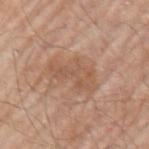Findings:
- biopsy status · catalogued during a skin exam; not biopsied
- lesion size · ≈5.5 mm
- body site · the arm
- subject · male, aged 63 to 67
- image source · ~15 mm tile from a whole-body skin photo
- automated metrics · an eccentricity of roughly 0.8 and two-axis asymmetry of about 0.45; an average lesion color of about L≈55 a*≈19 b*≈31 (CIELAB) and about 7 CIELAB-L* units darker than the surrounding skin